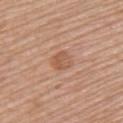Part of a total-body skin-imaging series; this lesion was reviewed on a skin check and was not flagged for biopsy.